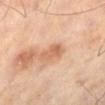<lesion>
  <biopsy_status>not biopsied; imaged during a skin examination</biopsy_status>
  <image>
    <source>total-body photography crop</source>
    <field_of_view_mm>15</field_of_view_mm>
  </image>
  <patient>
    <sex>male</sex>
    <age_approx>65</age_approx>
  </patient>
  <site>right lower leg</site>
</lesion>